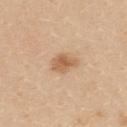The lesion was tiled from a total-body skin photograph and was not biopsied.
A close-up tile cropped from a whole-body skin photograph, about 15 mm across.
This is a white-light tile.
The patient is a female in their mid-20s.
Located on the upper back.
The lesion's longest dimension is about 3 mm.
An algorithmic analysis of the crop reported a lesion area of about 5 mm² and two-axis asymmetry of about 0.25. It also reported a mean CIELAB color near L≈60 a*≈20 b*≈36, roughly 11 lightness units darker than nearby skin, and a normalized lesion–skin contrast near 7.5.